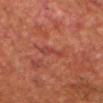No biopsy was performed on this lesion — it was imaged during a full skin examination and was not determined to be concerning. The tile uses cross-polarized illumination. This image is a 15 mm lesion crop taken from a total-body photograph. A male subject aged around 80. The lesion is located on the head or neck. About 3.5 mm across.